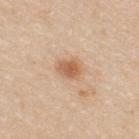Findings:
• follow-up · no biopsy performed (imaged during a skin exam)
• illumination · white-light
• patient · male, aged approximately 35
• imaging modality · 15 mm crop, total-body photography
• diameter · about 3 mm
• site · the upper back